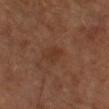workup = imaged on a skin check; not biopsied | patient = male, in their mid-60s | location = the left forearm | image = ~15 mm crop, total-body skin-cancer survey | tile lighting = cross-polarized | size = about 2.5 mm.